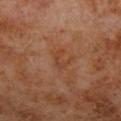Assessment:
This lesion was catalogued during total-body skin photography and was not selected for biopsy.
Background:
The subject is a male aged around 70. A 15 mm crop from a total-body photograph taken for skin-cancer surveillance. From the left lower leg. The total-body-photography lesion software estimated a footprint of about 3.5 mm², a shape eccentricity near 0.8, and a shape-asymmetry score of about 0.45 (0 = symmetric). The analysis additionally found a lesion color around L≈41 a*≈23 b*≈31 in CIELAB, roughly 6 lightness units darker than nearby skin, and a lesion-to-skin contrast of about 5 (normalized; higher = more distinct). It also reported border irregularity of about 5.5 on a 0–10 scale and peripheral color asymmetry of about 0. The analysis additionally found a classifier nevus-likeness of about 0/100 and a lesion-detection confidence of about 100/100. This is a cross-polarized tile. Measured at roughly 3 mm in maximum diameter.